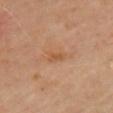biopsy status — imaged on a skin check; not biopsied | subject — female, in their 60s | body site — the chest | imaging modality — ~15 mm crop, total-body skin-cancer survey.